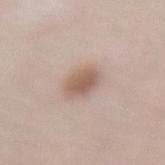Imaged during a routine full-body skin examination; the lesion was not biopsied and no histopathology is available. Measured at roughly 3 mm in maximum diameter. A region of skin cropped from a whole-body photographic capture, roughly 15 mm wide. Imaged with white-light lighting. Located on the back. A female patient aged approximately 65. Automated tile analysis of the lesion measured border irregularity of about 1.5 on a 0–10 scale, internal color variation of about 3 on a 0–10 scale, and radial color variation of about 1.